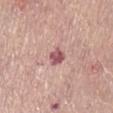{"biopsy_status": "not biopsied; imaged during a skin examination", "image": {"source": "total-body photography crop", "field_of_view_mm": 15}, "automated_metrics": {"cielab_L": 54, "cielab_a": 27, "cielab_b": 19, "vs_skin_darker_L": 14.0, "vs_skin_contrast_norm": 10.0, "border_irregularity_0_10": 2.0, "color_variation_0_10": 3.0}, "site": "abdomen", "lesion_size": {"long_diameter_mm_approx": 2.5}, "patient": {"sex": "female", "age_approx": 65}, "lighting": "white-light"}